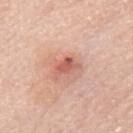Case summary:
- notes · catalogued during a skin exam; not biopsied
- image · 15 mm crop, total-body photography
- lesion size · ~3 mm (longest diameter)
- tile lighting · white-light
- subject · male, aged approximately 80
- anatomic site · the mid back